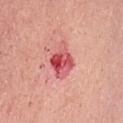The lesion was tiled from a total-body skin photograph and was not biopsied. An algorithmic analysis of the crop reported about 14 CIELAB-L* units darker than the surrounding skin. Longest diameter approximately 4 mm. The lesion is located on the abdomen. A close-up tile cropped from a whole-body skin photograph, about 15 mm across. Captured under white-light illumination. A female subject about 55 years old.